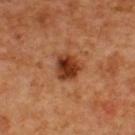Notes:
* notes — catalogued during a skin exam; not biopsied
* lesion diameter — ~3 mm (longest diameter)
* patient — male, aged 58 to 62
* tile lighting — cross-polarized
* body site — the back
* imaging modality — ~15 mm crop, total-body skin-cancer survey
* automated metrics — an eccentricity of roughly 0.5 and two-axis asymmetry of about 0.2; an average lesion color of about L≈34 a*≈25 b*≈33 (CIELAB), about 13 CIELAB-L* units darker than the surrounding skin, and a lesion-to-skin contrast of about 11.5 (normalized; higher = more distinct); a border-irregularity index near 2/10, a color-variation rating of about 5.5/10, and a peripheral color-asymmetry measure near 2; a classifier nevus-likeness of about 95/100 and a detector confidence of about 100 out of 100 that the crop contains a lesion